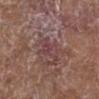Impression: The lesion was photographed on a routine skin check and not biopsied; there is no pathology result. Context: From the left lower leg. A region of skin cropped from a whole-body photographic capture, roughly 15 mm wide. A female patient aged 78 to 82.